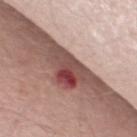biopsy status — total-body-photography surveillance lesion; no biopsy | illumination — white-light | patient — male, aged 63–67 | automated metrics — a mean CIELAB color near L≈46 a*≈23 b*≈22 and a normalized lesion–skin contrast near 12.5; border irregularity of about 6 on a 0–10 scale and peripheral color asymmetry of about 6; lesion-presence confidence of about 100/100 | lesion diameter — about 6 mm | acquisition — ~15 mm tile from a whole-body skin photo | location — the back.